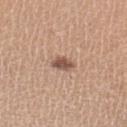- follow-up · catalogued during a skin exam; not biopsied
- image source · total-body-photography crop, ~15 mm field of view
- image-analysis metrics · an area of roughly 4.5 mm², an eccentricity of roughly 0.75, and a symmetry-axis asymmetry near 0.25; a border-irregularity rating of about 2.5/10, a within-lesion color-variation index near 2.5/10, and radial color variation of about 1
- lighting · white-light
- subject · female, aged approximately 35
- site · the left lower leg